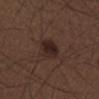Captured during whole-body skin photography for melanoma surveillance; the lesion was not biopsied.
A male patient approximately 50 years of age.
Imaged with white-light lighting.
A 15 mm crop from a total-body photograph taken for skin-cancer surveillance.
Located on the right lower leg.
Approximately 2.5 mm at its widest.